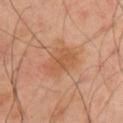Q: Who is the patient?
A: male, approximately 50 years of age
Q: Lesion location?
A: the chest
Q: What is the imaging modality?
A: ~15 mm crop, total-body skin-cancer survey
Q: Lesion size?
A: ≈5 mm